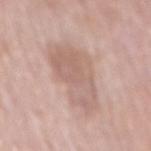Assessment:
Part of a total-body skin-imaging series; this lesion was reviewed on a skin check and was not flagged for biopsy.
Clinical summary:
A close-up tile cropped from a whole-body skin photograph, about 15 mm across. The patient is a male aged 73 to 77. The lesion is on the mid back.